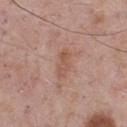Clinical impression:
The lesion was tiled from a total-body skin photograph and was not biopsied.
Background:
The patient is a male in their mid- to late 70s. Cropped from a total-body skin-imaging series; the visible field is about 15 mm. On the chest.